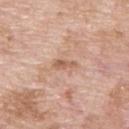  biopsy_status: not biopsied; imaged during a skin examination
  patient:
    sex: male
    age_approx: 60
  lesion_size:
    long_diameter_mm_approx: 3.0
  site: upper back
  image:
    source: total-body photography crop
    field_of_view_mm: 15